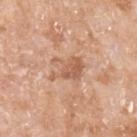Clinical impression:
The lesion was photographed on a routine skin check and not biopsied; there is no pathology result.
Context:
The recorded lesion diameter is about 3.5 mm. From the arm. A region of skin cropped from a whole-body photographic capture, roughly 15 mm wide. Captured under white-light illumination. A female patient, roughly 75 years of age.